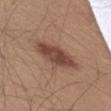Assessment: No biopsy was performed on this lesion — it was imaged during a full skin examination and was not determined to be concerning. Background: A male patient aged 58 to 62. The lesion is located on the left thigh. A close-up tile cropped from a whole-body skin photograph, about 15 mm across.